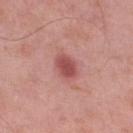<tbp_lesion>
  <biopsy_status>not biopsied; imaged during a skin examination</biopsy_status>
  <site>right lower leg</site>
  <image>
    <source>total-body photography crop</source>
    <field_of_view_mm>15</field_of_view_mm>
  </image>
  <patient>
    <sex>male</sex>
    <age_approx>55</age_approx>
  </patient>
</tbp_lesion>